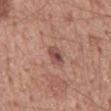biopsy_status: not biopsied; imaged during a skin examination
lesion_size:
  long_diameter_mm_approx: 3.0
patient:
  sex: male
  age_approx: 55
site: mid back
lighting: white-light
image:
  source: total-body photography crop
  field_of_view_mm: 15
automated_metrics:
  area_mm2_approx: 4.0
  eccentricity: 0.85
  shape_asymmetry: 0.2
  vs_skin_contrast_norm: 8.0
  border_irregularity_0_10: 2.5
  color_variation_0_10: 5.0
  peripheral_color_asymmetry: 1.5
  nevus_likeness_0_100: 35
  lesion_detection_confidence_0_100: 100